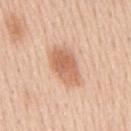Assessment:
This lesion was catalogued during total-body skin photography and was not selected for biopsy.
Clinical summary:
Cropped from a whole-body photographic skin survey; the tile spans about 15 mm. The lesion is on the mid back. About 5 mm across. The subject is a male aged approximately 60. This is a white-light tile.